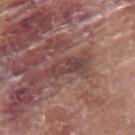Captured during whole-body skin photography for melanoma surveillance; the lesion was not biopsied.